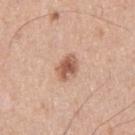workup: imaged on a skin check; not biopsied
size: ~3 mm (longest diameter)
image-analysis metrics: a mean CIELAB color near L≈57 a*≈22 b*≈30 and roughly 14 lightness units darker than nearby skin; a color-variation rating of about 4/10 and a peripheral color-asymmetry measure near 1.5
patient: male, approximately 45 years of age
body site: the right upper arm
image: ~15 mm tile from a whole-body skin photo